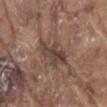The lesion was photographed on a routine skin check and not biopsied; there is no pathology result.
A male patient, in their 80s.
The lesion is on the front of the torso.
Imaged with white-light lighting.
About 4.5 mm across.
A lesion tile, about 15 mm wide, cut from a 3D total-body photograph.
The total-body-photography lesion software estimated an area of roughly 8 mm² and a shape eccentricity near 0.8. The analysis additionally found a nevus-likeness score of about 0/100 and lesion-presence confidence of about 95/100.